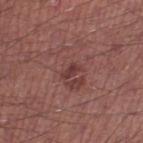workup — catalogued during a skin exam; not biopsied
location — the right thigh
tile lighting — white-light illumination
image source — ~15 mm crop, total-body skin-cancer survey
patient — male, in their mid-40s
automated metrics — two-axis asymmetry of about 0.35; an average lesion color of about L≈38 a*≈24 b*≈21 (CIELAB), a lesion–skin lightness drop of about 8, and a normalized border contrast of about 7; a border-irregularity index near 3.5/10, internal color variation of about 0 on a 0–10 scale, and peripheral color asymmetry of about 0; an automated nevus-likeness rating near 5 out of 100 and a detector confidence of about 100 out of 100 that the crop contains a lesion